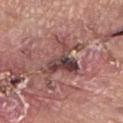<case>
<biopsy_status>not biopsied; imaged during a skin examination</biopsy_status>
<image>
  <source>total-body photography crop</source>
  <field_of_view_mm>15</field_of_view_mm>
</image>
<site>left lower leg</site>
<patient>
  <sex>female</sex>
  <age_approx>70</age_approx>
</patient>
</case>